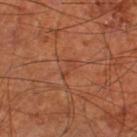Captured during whole-body skin photography for melanoma surveillance; the lesion was not biopsied.
A 15 mm crop from a total-body photograph taken for skin-cancer surveillance.
A male patient in their mid-60s.
Located on the left thigh.